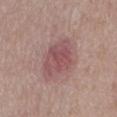workup: total-body-photography surveillance lesion; no biopsy
body site: the back
imaging modality: ~15 mm crop, total-body skin-cancer survey
patient: male, aged approximately 75